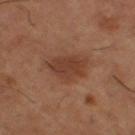Q: What is the anatomic site?
A: the right thigh
Q: Patient demographics?
A: male, roughly 65 years of age
Q: How was this image acquired?
A: 15 mm crop, total-body photography
Q: What is the lesion's diameter?
A: ~5 mm (longest diameter)
Q: Illumination type?
A: cross-polarized
Q: Automated lesion metrics?
A: an average lesion color of about L≈40 a*≈21 b*≈29 (CIELAB) and a normalized lesion–skin contrast near 7; a border-irregularity index near 2.5/10, internal color variation of about 2.5 on a 0–10 scale, and peripheral color asymmetry of about 1